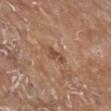follow-up = imaged on a skin check; not biopsied | image source = ~15 mm crop, total-body skin-cancer survey | size = about 2.5 mm | subject = female, in their mid-70s | body site = the right lower leg.